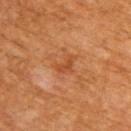A 15 mm close-up tile from a total-body photography series done for melanoma screening. A male patient, in their 60s. Located on the upper back.